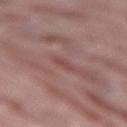workup: catalogued during a skin exam; not biopsied
TBP lesion metrics: an outline eccentricity of about 0.95 (0 = round, 1 = elongated) and a symmetry-axis asymmetry near 0.25; peripheral color asymmetry of about 0; a detector confidence of about 55 out of 100 that the crop contains a lesion
imaging modality: 15 mm crop, total-body photography
subject: male, aged approximately 40
tile lighting: white-light
size: about 2.5 mm
location: the leg